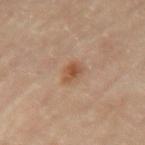Assessment:
Captured during whole-body skin photography for melanoma surveillance; the lesion was not biopsied.
Background:
On the left upper arm. The tile uses cross-polarized illumination. The patient is a female approximately 65 years of age. An algorithmic analysis of the crop reported a footprint of about 4 mm² and a shape eccentricity near 0.75. It also reported a peripheral color-asymmetry measure near 1. A lesion tile, about 15 mm wide, cut from a 3D total-body photograph.